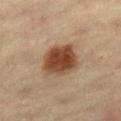Assessment: The lesion was photographed on a routine skin check and not biopsied; there is no pathology result. Context: Measured at roughly 4.5 mm in maximum diameter. This is a cross-polarized tile. A close-up tile cropped from a whole-body skin photograph, about 15 mm across. A male patient in their 60s. Located on the left thigh.